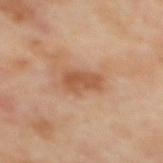The lesion was tiled from a total-body skin photograph and was not biopsied. An algorithmic analysis of the crop reported a footprint of about 7 mm², a shape eccentricity near 0.85, and two-axis asymmetry of about 0.25. The analysis additionally found a border-irregularity index near 3/10, a color-variation rating of about 2.5/10, and radial color variation of about 1. From the upper back. This image is a 15 mm lesion crop taken from a total-body photograph. A female subject in their 60s. Approximately 4 mm at its widest.